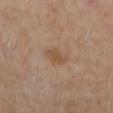Part of a total-body skin-imaging series; this lesion was reviewed on a skin check and was not flagged for biopsy. A female subject, aged around 50. The lesion is located on the left lower leg. A 15 mm crop from a total-body photograph taken for skin-cancer surveillance. Approximately 2.5 mm at its widest. Captured under cross-polarized illumination.